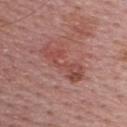Impression:
The lesion was photographed on a routine skin check and not biopsied; there is no pathology result.
Image and clinical context:
From the upper back. Cropped from a total-body skin-imaging series; the visible field is about 15 mm. The subject is a female in their mid- to late 50s.